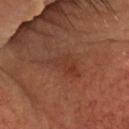Q: Is there a histopathology result?
A: no biopsy performed (imaged during a skin exam)
Q: What is the anatomic site?
A: the head or neck
Q: Who is the patient?
A: male, about 60 years old
Q: How was this image acquired?
A: ~15 mm crop, total-body skin-cancer survey
Q: How was the tile lit?
A: cross-polarized illumination
Q: How large is the lesion?
A: about 4.5 mm